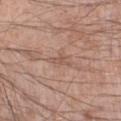Captured under white-light illumination.
The lesion is located on the left forearm.
A lesion tile, about 15 mm wide, cut from a 3D total-body photograph.
A male patient roughly 55 years of age.
The lesion's longest dimension is about 3 mm.
The total-body-photography lesion software estimated an average lesion color of about L≈54 a*≈19 b*≈26 (CIELAB) and a lesion–skin lightness drop of about 7. The analysis additionally found border irregularity of about 7 on a 0–10 scale, a within-lesion color-variation index near 0/10, and a peripheral color-asymmetry measure near 0.